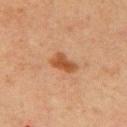biopsy status: imaged on a skin check; not biopsied | patient: male, in their 60s | size: ≈3 mm | anatomic site: the right upper arm | image source: total-body-photography crop, ~15 mm field of view | lighting: cross-polarized illumination | image-analysis metrics: a border-irregularity rating of about 3/10, internal color variation of about 2 on a 0–10 scale, and peripheral color asymmetry of about 0.5.